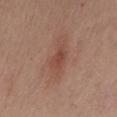follow-up: catalogued during a skin exam; not biopsied | subject: female, aged 48–52 | illumination: white-light illumination | image source: total-body-photography crop, ~15 mm field of view | automated lesion analysis: a classifier nevus-likeness of about 0/100 and a lesion-detection confidence of about 100/100 | diameter: ~3 mm (longest diameter) | site: the mid back.